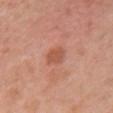follow-up: catalogued during a skin exam; not biopsied
image source: ~15 mm crop, total-body skin-cancer survey
body site: the front of the torso
patient: female, about 50 years old